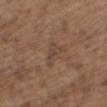| key | value |
|---|---|
| notes | catalogued during a skin exam; not biopsied |
| lesion size | ≈3.5 mm |
| patient | female, in their mid- to late 60s |
| imaging modality | 15 mm crop, total-body photography |
| location | the mid back |